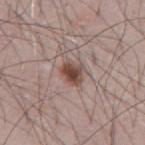Imaged during a routine full-body skin examination; the lesion was not biopsied and no histopathology is available.